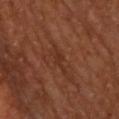{
  "biopsy_status": "not biopsied; imaged during a skin examination"
}